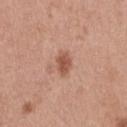Impression:
Recorded during total-body skin imaging; not selected for excision or biopsy.
Clinical summary:
A 15 mm close-up extracted from a 3D total-body photography capture. Longest diameter approximately 2.5 mm. An algorithmic analysis of the crop reported a border-irregularity index near 1.5/10 and a color-variation rating of about 2.5/10. The analysis additionally found a nevus-likeness score of about 90/100. The patient is a female about 40 years old. Located on the right thigh.